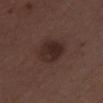Part of a total-body skin-imaging series; this lesion was reviewed on a skin check and was not flagged for biopsy. The subject is a male approximately 50 years of age. A close-up tile cropped from a whole-body skin photograph, about 15 mm across.